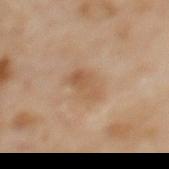TBP lesion metrics: a footprint of about 6 mm², an outline eccentricity of about 0.85 (0 = round, 1 = elongated), and two-axis asymmetry of about 0.25; an average lesion color of about L≈55 a*≈18 b*≈33 (CIELAB) and about 8 CIELAB-L* units darker than the surrounding skin; a border-irregularity index near 2.5/10, internal color variation of about 4 on a 0–10 scale, and radial color variation of about 1.5; a detector confidence of about 100 out of 100 that the crop contains a lesion | anatomic site: the upper back | illumination: cross-polarized illumination | acquisition: 15 mm crop, total-body photography | size: ~4 mm (longest diameter) | subject: female, aged approximately 60.